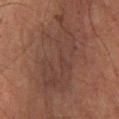This is a cross-polarized tile. Automated image analysis of the tile measured an area of roughly 28 mm², a shape eccentricity near 0.9, and a shape-asymmetry score of about 0.45 (0 = symmetric). And it measured radial color variation of about 1. It also reported a detector confidence of about 60 out of 100 that the crop contains a lesion. A close-up tile cropped from a whole-body skin photograph, about 15 mm across. The subject is a male aged approximately 65. On the right lower leg.